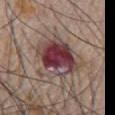Q: Is there a histopathology result?
A: catalogued during a skin exam; not biopsied
Q: Automated lesion metrics?
A: an area of roughly 19 mm², an eccentricity of roughly 0.6, and a shape-asymmetry score of about 0.2 (0 = symmetric); a classifier nevus-likeness of about 0/100
Q: What is the lesion's diameter?
A: ~5.5 mm (longest diameter)
Q: Lesion location?
A: the chest
Q: Patient demographics?
A: male, in their mid-50s
Q: How was this image acquired?
A: ~15 mm crop, total-body skin-cancer survey